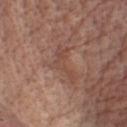Notes:
* workup — catalogued during a skin exam; not biopsied
* size — about 5 mm
* body site — the chest
* lighting — white-light
* image source — ~15 mm crop, total-body skin-cancer survey
* subject — male, aged 68 to 72